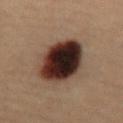| field | value |
|---|---|
| follow-up | catalogued during a skin exam; not biopsied |
| subject | female, aged 18–22 |
| location | the abdomen |
| image | total-body-photography crop, ~15 mm field of view |